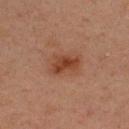notes: imaged on a skin check; not biopsied | patient: male, in their 30s | automated lesion analysis: a symmetry-axis asymmetry near 0.35; a border-irregularity index near 3.5/10 and peripheral color asymmetry of about 1; an automated nevus-likeness rating near 75 out of 100 and lesion-presence confidence of about 100/100 | imaging modality: total-body-photography crop, ~15 mm field of view | diameter: ≈4 mm | lighting: cross-polarized | location: the upper back.